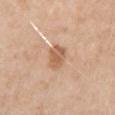From the right upper arm. A region of skin cropped from a whole-body photographic capture, roughly 15 mm wide. A male patient in their 70s. About 3 mm across. The tile uses white-light illumination.